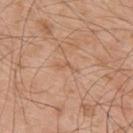Clinical impression: The lesion was tiled from a total-body skin photograph and was not biopsied. Clinical summary: Automated image analysis of the tile measured border irregularity of about 6.5 on a 0–10 scale, a within-lesion color-variation index near 0/10, and peripheral color asymmetry of about 0. The software also gave a detector confidence of about 95 out of 100 that the crop contains a lesion. Imaged with white-light lighting. A male patient aged 53 to 57. From the upper back. A 15 mm close-up extracted from a 3D total-body photography capture.